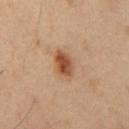No biopsy was performed on this lesion — it was imaged during a full skin examination and was not determined to be concerning. On the chest. The lesion-visualizer software estimated a symmetry-axis asymmetry near 0.2. And it measured a lesion color around L≈49 a*≈23 b*≈33 in CIELAB and a lesion–skin lightness drop of about 13. This is a cross-polarized tile. A 15 mm close-up extracted from a 3D total-body photography capture. A male subject, aged around 55.